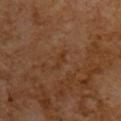Notes:
* follow-up: catalogued during a skin exam; not biopsied
* acquisition: total-body-photography crop, ~15 mm field of view
* subject: male, aged approximately 60
* body site: the upper back